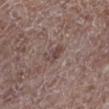Background:
A lesion tile, about 15 mm wide, cut from a 3D total-body photograph. Approximately 2.5 mm at its widest. From the left lower leg. A male subject aged approximately 70.